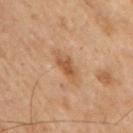Part of a total-body skin-imaging series; this lesion was reviewed on a skin check and was not flagged for biopsy. A 15 mm crop from a total-body photograph taken for skin-cancer surveillance. The lesion is on the right upper arm. A male patient aged 68 to 72. The lesion's longest dimension is about 3 mm. Captured under cross-polarized illumination.